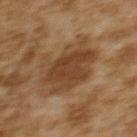No biopsy was performed on this lesion — it was imaged during a full skin examination and was not determined to be concerning.
A female subject, in their 60s.
The lesion is on the upper back.
Captured under cross-polarized illumination.
A 15 mm close-up tile from a total-body photography series done for melanoma screening.
Approximately 6.5 mm at its widest.